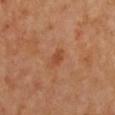Assessment: No biopsy was performed on this lesion — it was imaged during a full skin examination and was not determined to be concerning. Background: The lesion is on the chest. A region of skin cropped from a whole-body photographic capture, roughly 15 mm wide. A female subject roughly 45 years of age.